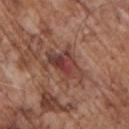The lesion was photographed on a routine skin check and not biopsied; there is no pathology result. The lesion is located on the front of the torso. The total-body-photography lesion software estimated a mean CIELAB color near L≈41 a*≈23 b*≈25. And it measured an automated nevus-likeness rating near 0 out of 100. Captured under white-light illumination. A male patient, aged 68–72. This image is a 15 mm lesion crop taken from a total-body photograph.